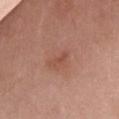The patient is a female about 40 years old.
The total-body-photography lesion software estimated border irregularity of about 5 on a 0–10 scale, internal color variation of about 0 on a 0–10 scale, and a peripheral color-asymmetry measure near 0.
About 2.5 mm across.
A 15 mm close-up tile from a total-body photography series done for melanoma screening.
The lesion is on the chest.
This is a white-light tile.